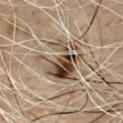Case summary:
* workup: imaged on a skin check; not biopsied
* tile lighting: cross-polarized illumination
* body site: the chest
* image source: total-body-photography crop, ~15 mm field of view
* TBP lesion metrics: a footprint of about 22 mm² and a symmetry-axis asymmetry near 0.4; a mean CIELAB color near L≈37 a*≈10 b*≈22, about 11 CIELAB-L* units darker than the surrounding skin, and a lesion-to-skin contrast of about 9.5 (normalized; higher = more distinct); border irregularity of about 6.5 on a 0–10 scale and radial color variation of about 4.5; an automated nevus-likeness rating near 15 out of 100
* lesion size: ~6 mm (longest diameter)
* patient: male, about 50 years old